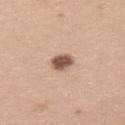Findings:
* notes · no biopsy performed (imaged during a skin exam)
* imaging modality · ~15 mm crop, total-body skin-cancer survey
* site · the left upper arm
* illumination · white-light
* size · about 2.5 mm
* subject · female, aged 23 to 27